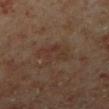notes = total-body-photography surveillance lesion; no biopsy.